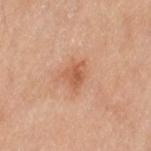About 3 mm across. A female subject aged around 60. A lesion tile, about 15 mm wide, cut from a 3D total-body photograph. The lesion is on the left thigh. Imaged with cross-polarized lighting.